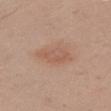patient: female, approximately 30 years of age
lesion size: about 4.5 mm
tile lighting: white-light
imaging modality: 15 mm crop, total-body photography
location: the right thigh